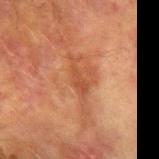{
  "biopsy_status": "not biopsied; imaged during a skin examination",
  "site": "right forearm",
  "image": {
    "source": "total-body photography crop",
    "field_of_view_mm": 15
  },
  "lighting": "cross-polarized",
  "patient": {
    "sex": "male",
    "age_approx": 75
  },
  "lesion_size": {
    "long_diameter_mm_approx": 4.0
  }
}